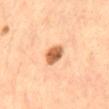The lesion was tiled from a total-body skin photograph and was not biopsied. This is a cross-polarized tile. About 3 mm across. The lesion is on the abdomen. A 15 mm crop from a total-body photograph taken for skin-cancer surveillance. A female subject, in their mid-60s.